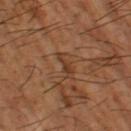subject — male, aged 63 to 67 | body site — the leg | image source — ~15 mm crop, total-body skin-cancer survey.